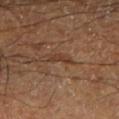<case>
<biopsy_status>not biopsied; imaged during a skin examination</biopsy_status>
<image>
  <source>total-body photography crop</source>
  <field_of_view_mm>15</field_of_view_mm>
</image>
<automated_metrics>
  <area_mm2_approx>2.5</area_mm2_approx>
  <eccentricity>0.9</eccentricity>
  <shape_asymmetry>0.3</shape_asymmetry>
  <cielab_L>33</cielab_L>
  <cielab_a>17</cielab_a>
  <cielab_b>26</cielab_b>
  <vs_skin_darker_L>6.0</vs_skin_darker_L>
  <vs_skin_contrast_norm>6.5</vs_skin_contrast_norm>
</automated_metrics>
<patient>
  <sex>male</sex>
  <age_approx>60</age_approx>
</patient>
<site>right lower leg</site>
</case>